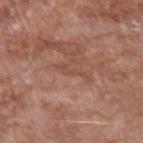Imaged during a routine full-body skin examination; the lesion was not biopsied and no histopathology is available.
From the left upper arm.
Longest diameter approximately 2.5 mm.
A male patient aged approximately 60.
A roughly 15 mm field-of-view crop from a total-body skin photograph.
Imaged with white-light lighting.